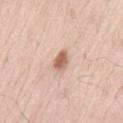Notes:
* biopsy status · no biopsy performed (imaged during a skin exam)
* subject · male, approximately 55 years of age
* acquisition · ~15 mm crop, total-body skin-cancer survey
* site · the lower back